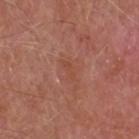workup: total-body-photography surveillance lesion; no biopsy
automated metrics: a footprint of about 2 mm² and an outline eccentricity of about 0.9 (0 = round, 1 = elongated); an average lesion color of about L≈47 a*≈25 b*≈31 (CIELAB); a border-irregularity index near 4.5/10, a color-variation rating of about 0/10, and a peripheral color-asymmetry measure near 0; a classifier nevus-likeness of about 0/100 and lesion-presence confidence of about 100/100
imaging modality: ~15 mm crop, total-body skin-cancer survey
diameter: about 2.5 mm
lighting: white-light
location: the head or neck
subject: male, in their mid- to late 50s